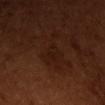| field | value |
|---|---|
| follow-up | imaged on a skin check; not biopsied |
| anatomic site | the chest |
| image | total-body-photography crop, ~15 mm field of view |
| lesion diameter | ≈2.5 mm |
| tile lighting | cross-polarized illumination |
| subject | female, aged approximately 55 |